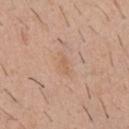Impression: Part of a total-body skin-imaging series; this lesion was reviewed on a skin check and was not flagged for biopsy. Image and clinical context: This is a white-light tile. From the chest. The lesion's longest dimension is about 3 mm. An algorithmic analysis of the crop reported a footprint of about 3 mm², an outline eccentricity of about 0.85 (0 = round, 1 = elongated), and two-axis asymmetry of about 0.35. The software also gave a lesion color around L≈61 a*≈19 b*≈32 in CIELAB, a lesion–skin lightness drop of about 6, and a lesion-to-skin contrast of about 4.5 (normalized; higher = more distinct). The analysis additionally found a border-irregularity index near 4/10, internal color variation of about 1.5 on a 0–10 scale, and peripheral color asymmetry of about 0.5. A 15 mm crop from a total-body photograph taken for skin-cancer surveillance. The subject is a male aged 38–42.